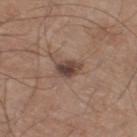This lesion was catalogued during total-body skin photography and was not selected for biopsy. A roughly 15 mm field-of-view crop from a total-body skin photograph. Imaged with white-light lighting. The patient is a male in their 60s. An algorithmic analysis of the crop reported an area of roughly 5 mm², an eccentricity of roughly 0.75, and a symmetry-axis asymmetry near 0.25. The software also gave roughly 12 lightness units darker than nearby skin. It also reported a nevus-likeness score of about 70/100 and a detector confidence of about 100 out of 100 that the crop contains a lesion. The lesion is on the right thigh. Approximately 3 mm at its widest.